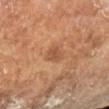Clinical impression:
This lesion was catalogued during total-body skin photography and was not selected for biopsy.
Background:
A 15 mm close-up tile from a total-body photography series done for melanoma screening. The subject is a male aged around 65. Measured at roughly 2.5 mm in maximum diameter.